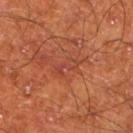{
  "biopsy_status": "not biopsied; imaged during a skin examination",
  "site": "leg",
  "automated_metrics": {
    "cielab_L": 42,
    "cielab_a": 29,
    "cielab_b": 31,
    "vs_skin_darker_L": 5.0,
    "vs_skin_contrast_norm": 5.0,
    "border_irregularity_0_10": 5.5,
    "peripheral_color_asymmetry": 0.0
  },
  "lighting": "cross-polarized",
  "patient": {
    "sex": "male",
    "age_approx": 65
  },
  "image": {
    "source": "total-body photography crop",
    "field_of_view_mm": 15
  },
  "lesion_size": {
    "long_diameter_mm_approx": 3.0
  }
}